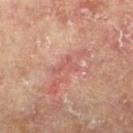The lesion was photographed on a routine skin check and not biopsied; there is no pathology result. The subject is a female aged around 75. The tile uses cross-polarized illumination. From the right lower leg. A close-up tile cropped from a whole-body skin photograph, about 15 mm across.